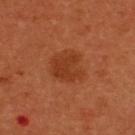Q: Is there a histopathology result?
A: imaged on a skin check; not biopsied
Q: Automated lesion metrics?
A: a nevus-likeness score of about 95/100 and lesion-presence confidence of about 100/100
Q: What is the imaging modality?
A: ~15 mm crop, total-body skin-cancer survey
Q: How was the tile lit?
A: cross-polarized
Q: Patient demographics?
A: female, aged approximately 50
Q: What is the lesion's diameter?
A: about 4.5 mm